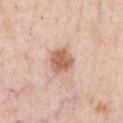biopsy_status: not biopsied; imaged during a skin examination
image:
  source: total-body photography crop
  field_of_view_mm: 15
site: chest
patient:
  sex: male
  age_approx: 50